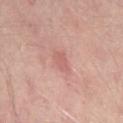Assessment: Part of a total-body skin-imaging series; this lesion was reviewed on a skin check and was not flagged for biopsy. Background: Located on the lower back. The tile uses cross-polarized illumination. The total-body-photography lesion software estimated an eccentricity of roughly 0.8 and two-axis asymmetry of about 0.25. The analysis additionally found a lesion–skin lightness drop of about 7. The patient is about 55 years old. This image is a 15 mm lesion crop taken from a total-body photograph.